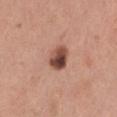This lesion was catalogued during total-body skin photography and was not selected for biopsy.
A female subject, aged 28 to 32.
A close-up tile cropped from a whole-body skin photograph, about 15 mm across.
An algorithmic analysis of the crop reported a lesion area of about 6.5 mm², an eccentricity of roughly 0.55, and a shape-asymmetry score of about 0.15 (0 = symmetric). The analysis additionally found a border-irregularity rating of about 1.5/10, a within-lesion color-variation index near 9.5/10, and radial color variation of about 3.5. And it measured a nevus-likeness score of about 60/100 and a lesion-detection confidence of about 100/100.
On the leg.